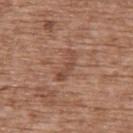Notes:
– biopsy status · catalogued during a skin exam; not biopsied
– lesion diameter · about 4 mm
– acquisition · ~15 mm tile from a whole-body skin photo
– body site · the upper back
– TBP lesion metrics · a color-variation rating of about 1.5/10 and peripheral color asymmetry of about 0.5; lesion-presence confidence of about 95/100
– patient · female, in their mid- to late 70s